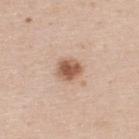notes = total-body-photography surveillance lesion; no biopsy
lesion diameter = ~2.5 mm (longest diameter)
subject = male, in their mid- to late 40s
acquisition = ~15 mm crop, total-body skin-cancer survey
illumination = white-light
anatomic site = the upper back